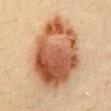Part of a total-body skin-imaging series; this lesion was reviewed on a skin check and was not flagged for biopsy.
The lesion is on the mid back.
Measured at roughly 9 mm in maximum diameter.
The patient is a female roughly 40 years of age.
Imaged with cross-polarized lighting.
A 15 mm close-up extracted from a 3D total-body photography capture.